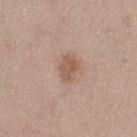Findings:
– biopsy status · catalogued during a skin exam; not biopsied
– subject · female, aged 38–42
– lesion diameter · about 3 mm
– imaging modality · ~15 mm tile from a whole-body skin photo
– anatomic site · the left thigh
– automated lesion analysis · border irregularity of about 2.5 on a 0–10 scale and internal color variation of about 2 on a 0–10 scale; a detector confidence of about 100 out of 100 that the crop contains a lesion
– lighting · white-light illumination